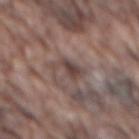follow-up: no biopsy performed (imaged during a skin exam)
body site: the mid back
image-analysis metrics: a lesion area of about 5 mm², an eccentricity of roughly 0.7, and a shape-asymmetry score of about 0.45 (0 = symmetric); a classifier nevus-likeness of about 5/100 and a lesion-detection confidence of about 80/100
patient: male, aged 73–77
acquisition: 15 mm crop, total-body photography
tile lighting: white-light
size: ~3 mm (longest diameter)